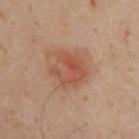Q: Was this lesion biopsied?
A: imaged on a skin check; not biopsied